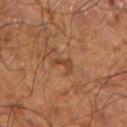image-analysis metrics = an area of roughly 3 mm² and an eccentricity of roughly 0.9; a lesion color around L≈35 a*≈19 b*≈29 in CIELAB and roughly 6 lightness units darker than nearby skin; border irregularity of about 5.5 on a 0–10 scale; an automated nevus-likeness rating near 0 out of 100 and a lesion-detection confidence of about 100/100
size = ~2.5 mm (longest diameter)
patient = male, roughly 70 years of age
acquisition = ~15 mm tile from a whole-body skin photo
illumination = cross-polarized illumination
body site = the left forearm